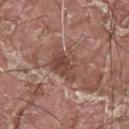Part of a total-body skin-imaging series; this lesion was reviewed on a skin check and was not flagged for biopsy.
On the upper back.
A male subject aged 38–42.
Cropped from a whole-body photographic skin survey; the tile spans about 15 mm.
This is a white-light tile.